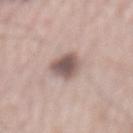Assessment:
Part of a total-body skin-imaging series; this lesion was reviewed on a skin check and was not flagged for biopsy.
Acquisition and patient details:
The patient is a male in their mid-60s. Measured at roughly 4 mm in maximum diameter. A lesion tile, about 15 mm wide, cut from a 3D total-body photograph. Imaged with white-light lighting. Located on the mid back.